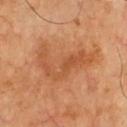Q: What did automated image analysis measure?
A: an area of roughly 14 mm², a shape eccentricity near 0.85, and a symmetry-axis asymmetry near 0.6; an average lesion color of about L≈55 a*≈27 b*≈40 (CIELAB) and about 8 CIELAB-L* units darker than the surrounding skin
Q: How large is the lesion?
A: about 6.5 mm
Q: Lesion location?
A: the chest
Q: What kind of image is this?
A: total-body-photography crop, ~15 mm field of view
Q: What lighting was used for the tile?
A: cross-polarized